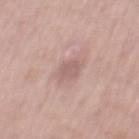Impression: Imaged during a routine full-body skin examination; the lesion was not biopsied and no histopathology is available. Background: This is a white-light tile. This image is a 15 mm lesion crop taken from a total-body photograph. Located on the mid back. A male subject about 55 years old.